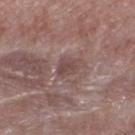{
  "biopsy_status": "not biopsied; imaged during a skin examination",
  "patient": {
    "sex": "male",
    "age_approx": 55
  },
  "image": {
    "source": "total-body photography crop",
    "field_of_view_mm": 15
  },
  "site": "left lower leg",
  "lighting": "white-light",
  "lesion_size": {
    "long_diameter_mm_approx": 2.5
  },
  "automated_metrics": {
    "border_irregularity_0_10": 3.0,
    "peripheral_color_asymmetry": 1.0,
    "nevus_likeness_0_100": 0
  }
}